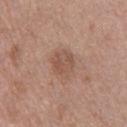The lesion was tiled from a total-body skin photograph and was not biopsied.
This is a white-light tile.
Cropped from a whole-body photographic skin survey; the tile spans about 15 mm.
A female subject, roughly 40 years of age.
An algorithmic analysis of the crop reported a footprint of about 7.5 mm² and an eccentricity of roughly 0.6. It also reported an average lesion color of about L≈52 a*≈20 b*≈27 (CIELAB), roughly 8 lightness units darker than nearby skin, and a normalized lesion–skin contrast near 6. The software also gave a color-variation rating of about 3/10 and radial color variation of about 1.
The recorded lesion diameter is about 3.5 mm.
From the right thigh.